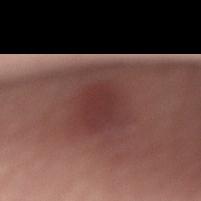Impression: The lesion was photographed on a routine skin check and not biopsied; there is no pathology result. Image and clinical context: A 15 mm close-up tile from a total-body photography series done for melanoma screening. This is a white-light tile. A male subject, aged approximately 70. The lesion is located on the lower back.